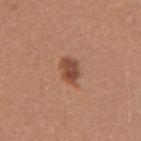follow-up — no biopsy performed (imaged during a skin exam)
patient — female, aged 18–22
acquisition — 15 mm crop, total-body photography
automated lesion analysis — a border-irregularity rating of about 2.5/10 and peripheral color asymmetry of about 1; a detector confidence of about 100 out of 100 that the crop contains a lesion
location — the right upper arm
diameter — ~3 mm (longest diameter)
tile lighting — white-light